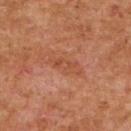Part of a total-body skin-imaging series; this lesion was reviewed on a skin check and was not flagged for biopsy. A female subject about 60 years old. An algorithmic analysis of the crop reported a mean CIELAB color near L≈43 a*≈24 b*≈30 and a normalized lesion–skin contrast near 5. From the upper back. Captured under cross-polarized illumination. Longest diameter approximately 4 mm. Cropped from a whole-body photographic skin survey; the tile spans about 15 mm.